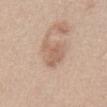{
  "biopsy_status": "not biopsied; imaged during a skin examination",
  "automated_metrics": {
    "cielab_L": 61,
    "cielab_a": 19,
    "cielab_b": 28,
    "vs_skin_darker_L": 8.0,
    "vs_skin_contrast_norm": 5.5,
    "border_irregularity_0_10": 5.0,
    "color_variation_0_10": 2.0,
    "peripheral_color_asymmetry": 0.5,
    "nevus_likeness_0_100": 10
  },
  "image": {
    "source": "total-body photography crop",
    "field_of_view_mm": 15
  },
  "patient": {
    "sex": "female",
    "age_approx": 40
  },
  "lesion_size": {
    "long_diameter_mm_approx": 4.0
  },
  "site": "abdomen"
}